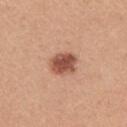workup: catalogued during a skin exam; not biopsied | site: the right upper arm | subject: female, aged around 45 | size: about 3 mm | tile lighting: white-light illumination | image: 15 mm crop, total-body photography.